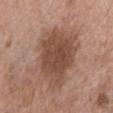Q: Was a biopsy performed?
A: imaged on a skin check; not biopsied
Q: How was the tile lit?
A: white-light illumination
Q: Where on the body is the lesion?
A: the chest
Q: What is the lesion's diameter?
A: ~8 mm (longest diameter)
Q: Patient demographics?
A: male, in their 50s
Q: What kind of image is this?
A: total-body-photography crop, ~15 mm field of view
Q: Automated lesion metrics?
A: a footprint of about 30 mm² and an eccentricity of roughly 0.8; an average lesion color of about L≈48 a*≈19 b*≈28 (CIELAB), about 12 CIELAB-L* units darker than the surrounding skin, and a normalized lesion–skin contrast near 8.5; a border-irregularity rating of about 3/10, a within-lesion color-variation index near 3/10, and radial color variation of about 1; an automated nevus-likeness rating near 20 out of 100